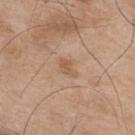From the mid back.
Cropped from a total-body skin-imaging series; the visible field is about 15 mm.
A male subject, aged 73–77.